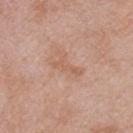biopsy status: total-body-photography surveillance lesion; no biopsy | lesion size: about 4 mm | location: the right upper arm | patient: male, roughly 55 years of age | acquisition: ~15 mm crop, total-body skin-cancer survey | illumination: white-light.